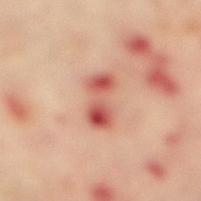{"biopsy_status": "not biopsied; imaged during a skin examination"}